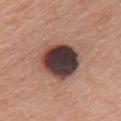Clinical impression: The lesion was photographed on a routine skin check and not biopsied; there is no pathology result. Context: The lesion's longest dimension is about 5 mm. The total-body-photography lesion software estimated a lesion color around L≈38 a*≈17 b*≈19 in CIELAB and about 23 CIELAB-L* units darker than the surrounding skin. And it measured a border-irregularity index near 1.5/10, a within-lesion color-variation index near 8.5/10, and peripheral color asymmetry of about 2.5. And it measured a nevus-likeness score of about 0/100 and a lesion-detection confidence of about 100/100. A 15 mm close-up tile from a total-body photography series done for melanoma screening. The lesion is located on the chest. A male subject, aged 58–62.